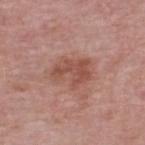The lesion was photographed on a routine skin check and not biopsied; there is no pathology result. Approximately 4.5 mm at its widest. A male patient, approximately 65 years of age. A lesion tile, about 15 mm wide, cut from a 3D total-body photograph. The lesion is on the upper back. Imaged with white-light lighting.